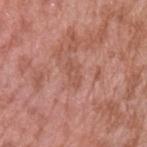follow-up: total-body-photography surveillance lesion; no biopsy | subject: male, aged 38–42 | tile lighting: white-light | acquisition: ~15 mm crop, total-body skin-cancer survey | body site: the left upper arm | diameter: about 3 mm.